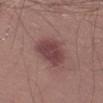- biopsy status · catalogued during a skin exam; not biopsied
- TBP lesion metrics · a lesion color around L≈43 a*≈23 b*≈18 in CIELAB, roughly 11 lightness units darker than nearby skin, and a normalized border contrast of about 9; a border-irregularity index near 2/10
- imaging modality · 15 mm crop, total-body photography
- lesion size · ~5 mm (longest diameter)
- anatomic site · the left thigh
- patient · male, aged approximately 55
- illumination · white-light